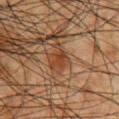This lesion was catalogued during total-body skin photography and was not selected for biopsy.
This is a cross-polarized tile.
The lesion's longest dimension is about 2.5 mm.
A region of skin cropped from a whole-body photographic capture, roughly 15 mm wide.
The lesion is on the chest.
The patient is a male in their 50s.